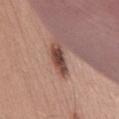Assessment:
The lesion was photographed on a routine skin check and not biopsied; there is no pathology result.
Clinical summary:
Imaged with white-light lighting. The subject is a male aged around 55. A 15 mm close-up extracted from a 3D total-body photography capture. Longest diameter approximately 4.5 mm. On the front of the torso.